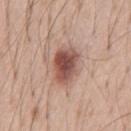Impression:
The lesion was photographed on a routine skin check and not biopsied; there is no pathology result.
Acquisition and patient details:
The lesion-visualizer software estimated a lesion area of about 11 mm², an eccentricity of roughly 0.75, and a shape-asymmetry score of about 0.2 (0 = symmetric). The software also gave a lesion color around L≈51 a*≈22 b*≈25 in CIELAB, about 15 CIELAB-L* units darker than the surrounding skin, and a normalized lesion–skin contrast near 10.5. It also reported a border-irregularity index near 2/10, internal color variation of about 6 on a 0–10 scale, and peripheral color asymmetry of about 1.5. And it measured an automated nevus-likeness rating near 95 out of 100 and a detector confidence of about 100 out of 100 that the crop contains a lesion. Located on the chest. Measured at roughly 4.5 mm in maximum diameter. A close-up tile cropped from a whole-body skin photograph, about 15 mm across. The tile uses white-light illumination. The patient is a male aged approximately 55.